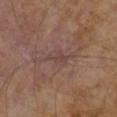notes=no biopsy performed (imaged during a skin exam); illumination=cross-polarized; lesion diameter=≈2.5 mm; subject=male, about 65 years old; imaging modality=total-body-photography crop, ~15 mm field of view.